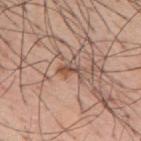workup=imaged on a skin check; not biopsied | image-analysis metrics=a border-irregularity index near 5/10, a within-lesion color-variation index near 4/10, and a peripheral color-asymmetry measure near 1 | anatomic site=the back | subject=male, in their mid- to late 50s | diameter=about 3 mm | acquisition=~15 mm tile from a whole-body skin photo.